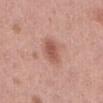Recorded during total-body skin imaging; not selected for excision or biopsy. This image is a 15 mm lesion crop taken from a total-body photograph. An algorithmic analysis of the crop reported a lesion area of about 7 mm², an outline eccentricity of about 0.75 (0 = round, 1 = elongated), and a symmetry-axis asymmetry near 0.2. The analysis additionally found a mean CIELAB color near L≈55 a*≈24 b*≈27, a lesion–skin lightness drop of about 10, and a normalized lesion–skin contrast near 7. And it measured a border-irregularity index near 2/10 and a color-variation rating of about 2.5/10. It also reported a classifier nevus-likeness of about 85/100 and lesion-presence confidence of about 100/100. From the abdomen. The tile uses white-light illumination. A female subject approximately 40 years of age. About 3.5 mm across.